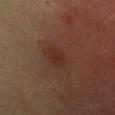follow-up: total-body-photography surveillance lesion; no biopsy | site: the chest | subject: female, aged 38–42 | lesion diameter: ~2.5 mm (longest diameter) | illumination: cross-polarized illumination | image: ~15 mm crop, total-body skin-cancer survey.